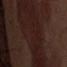– size: about 15 mm
– patient: male, aged 68 to 72
– image: 15 mm crop, total-body photography
– anatomic site: the abdomen
– illumination: white-light